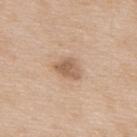biopsy status — no biopsy performed (imaged during a skin exam); automated lesion analysis — a footprint of about 6 mm², an outline eccentricity of about 0.7 (0 = round, 1 = elongated), and a shape-asymmetry score of about 0.3 (0 = symmetric); body site — the upper back; patient — male, approximately 65 years of age; diameter — ~3 mm (longest diameter); image source — total-body-photography crop, ~15 mm field of view; tile lighting — white-light illumination.